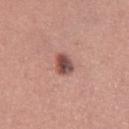Acquisition and patient details:
A female patient, approximately 30 years of age. A roughly 15 mm field-of-view crop from a total-body skin photograph. On the right thigh. An algorithmic analysis of the crop reported a border-irregularity rating of about 2/10, internal color variation of about 6 on a 0–10 scale, and peripheral color asymmetry of about 2.